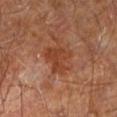follow-up: imaged on a skin check; not biopsied | TBP lesion metrics: an area of roughly 9.5 mm², a shape eccentricity near 0.3, and two-axis asymmetry of about 0.45; border irregularity of about 4.5 on a 0–10 scale, a color-variation rating of about 3/10, and peripheral color asymmetry of about 1 | acquisition: 15 mm crop, total-body photography | lighting: cross-polarized | anatomic site: the right leg | subject: male, approximately 60 years of age | size: ~4 mm (longest diameter).